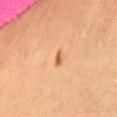notes=total-body-photography surveillance lesion; no biopsy
image source=15 mm crop, total-body photography
body site=the left thigh
patient=female, in their mid- to late 50s